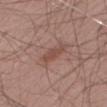  biopsy_status: not biopsied; imaged during a skin examination
  patient:
    sex: male
    age_approx: 60
  lighting: white-light
  image:
    source: total-body photography crop
    field_of_view_mm: 15
  lesion_size:
    long_diameter_mm_approx: 4.0
  site: right thigh